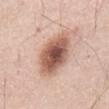Q: Who is the patient?
A: male, aged 53–57
Q: Where on the body is the lesion?
A: the abdomen
Q: What kind of image is this?
A: 15 mm crop, total-body photography